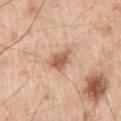<lesion>
  <biopsy_status>not biopsied; imaged during a skin examination</biopsy_status>
  <image>
    <source>total-body photography crop</source>
    <field_of_view_mm>15</field_of_view_mm>
  </image>
  <patient>
    <sex>male</sex>
    <age_approx>70</age_approx>
  </patient>
  <lesion_size>
    <long_diameter_mm_approx>3.0</long_diameter_mm_approx>
  </lesion_size>
  <lighting>white-light</lighting>
  <site>left upper arm</site>
</lesion>